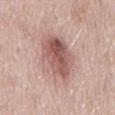Clinical impression: Captured during whole-body skin photography for melanoma surveillance; the lesion was not biopsied. Background: An algorithmic analysis of the crop reported an average lesion color of about L≈57 a*≈21 b*≈22 (CIELAB), a lesion–skin lightness drop of about 13, and a lesion-to-skin contrast of about 8.5 (normalized; higher = more distinct). The analysis additionally found a detector confidence of about 100 out of 100 that the crop contains a lesion. The lesion's longest dimension is about 7 mm. On the mid back. This image is a 15 mm lesion crop taken from a total-body photograph. Imaged with white-light lighting. A male subject, about 55 years old.